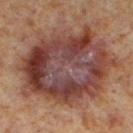biopsy status: total-body-photography surveillance lesion; no biopsy
patient: male, in their 60s
anatomic site: the left lower leg
illumination: cross-polarized illumination
acquisition: total-body-photography crop, ~15 mm field of view